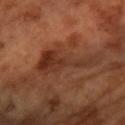{"biopsy_status": "not biopsied; imaged during a skin examination"}